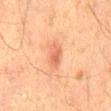follow-up — imaged on a skin check; not biopsied | image — ~15 mm tile from a whole-body skin photo | anatomic site — the mid back | size — about 3 mm | image-analysis metrics — a border-irregularity rating of about 2/10, a color-variation rating of about 3/10, and radial color variation of about 1; an automated nevus-likeness rating near 80 out of 100 and lesion-presence confidence of about 100/100 | lighting — cross-polarized illumination | patient — male, aged 63–67.